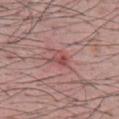Findings:
- biopsy status — imaged on a skin check; not biopsied
- imaging modality — 15 mm crop, total-body photography
- diameter — ≈2.5 mm
- patient — male, about 30 years old
- location — the chest
- automated metrics — border irregularity of about 4 on a 0–10 scale and a color-variation rating of about 3.5/10; a nevus-likeness score of about 5/100 and a lesion-detection confidence of about 100/100